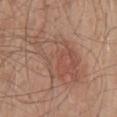The lesion was tiled from a total-body skin photograph and was not biopsied. A close-up tile cropped from a whole-body skin photograph, about 15 mm across. The lesion is located on the mid back. A male subject about 50 years old.